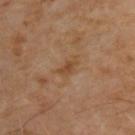Clinical impression: The lesion was photographed on a routine skin check and not biopsied; there is no pathology result. Clinical summary: Captured under cross-polarized illumination. A 15 mm close-up extracted from a 3D total-body photography capture. On the upper back. A male subject approximately 65 years of age.